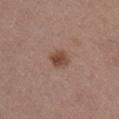Clinical impression:
This lesion was catalogued during total-body skin photography and was not selected for biopsy.
Background:
About 2.5 mm across. A lesion tile, about 15 mm wide, cut from a 3D total-body photograph. The lesion is on the right upper arm. The patient is a male about 40 years old.